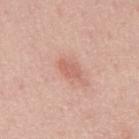Captured during whole-body skin photography for melanoma surveillance; the lesion was not biopsied. The lesion's longest dimension is about 2.5 mm. A male subject aged approximately 45. A close-up tile cropped from a whole-body skin photograph, about 15 mm across. The lesion is on the mid back.